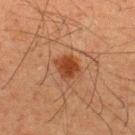This lesion was catalogued during total-body skin photography and was not selected for biopsy. Approximately 3 mm at its widest. From the upper back. A 15 mm close-up tile from a total-body photography series done for melanoma screening. A male patient, aged 33 to 37. The tile uses cross-polarized illumination.